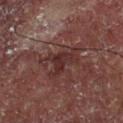Assessment:
This lesion was catalogued during total-body skin photography and was not selected for biopsy.
Context:
A 15 mm close-up tile from a total-body photography series done for melanoma screening. Longest diameter approximately 3.5 mm. The lesion is located on the chest. A male subject, about 55 years old. Automated tile analysis of the lesion measured a lesion area of about 4.5 mm² and an eccentricity of roughly 0.8. It also reported an average lesion color of about L≈25 a*≈19 b*≈18 (CIELAB), about 7 CIELAB-L* units darker than the surrounding skin, and a lesion-to-skin contrast of about 7.5 (normalized; higher = more distinct). It also reported border irregularity of about 5 on a 0–10 scale and peripheral color asymmetry of about 0.5. And it measured an automated nevus-likeness rating near 0 out of 100 and lesion-presence confidence of about 75/100.